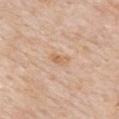The lesion was tiled from a total-body skin photograph and was not biopsied. A 15 mm close-up extracted from a 3D total-body photography capture. The patient is a male aged approximately 85 or older. From the mid back. This is a white-light tile.